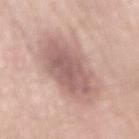The lesion was tiled from a total-body skin photograph and was not biopsied.
The lesion is located on the back.
The lesion's longest dimension is about 9.5 mm.
A lesion tile, about 15 mm wide, cut from a 3D total-body photograph.
A male patient approximately 70 years of age.
This is a white-light tile.